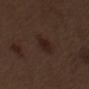The lesion was photographed on a routine skin check and not biopsied; there is no pathology result.